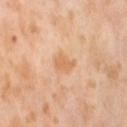| key | value |
|---|---|
| lesion diameter | ≈3 mm |
| illumination | cross-polarized illumination |
| subject | female, in their mid-50s |
| image-analysis metrics | an area of roughly 3.5 mm² and an eccentricity of roughly 0.8; a normalized lesion–skin contrast near 6.5 |
| body site | the right thigh |
| acquisition | ~15 mm crop, total-body skin-cancer survey |